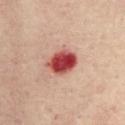biopsy status — catalogued during a skin exam; not biopsied | lesion size — ~4 mm (longest diameter) | location — the chest | illumination — cross-polarized illumination | subject — female, aged 43–47 | acquisition — ~15 mm crop, total-body skin-cancer survey.